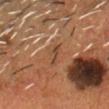biopsy status — catalogued during a skin exam; not biopsied | patient — male, in their mid-50s | lesion size — ≈3 mm | location — the head or neck | lighting — cross-polarized | image — ~15 mm tile from a whole-body skin photo.